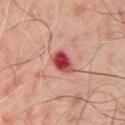  biopsy_status: not biopsied; imaged during a skin examination
  automated_metrics:
    cielab_L: 49
    cielab_a: 36
    cielab_b: 27
    vs_skin_darker_L: 17.0
    vs_skin_contrast_norm: 11.0
    border_irregularity_0_10: 2.0
    color_variation_0_10: 9.5
    peripheral_color_asymmetry: 3.0
    nevus_likeness_0_100: 0
  lesion_size:
    long_diameter_mm_approx: 3.0
  site: front of the torso
  image:
    source: total-body photography crop
    field_of_view_mm: 15
  lighting: cross-polarized
  patient:
    sex: male
    age_approx: 50